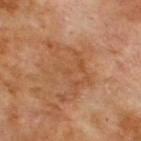workup: catalogued during a skin exam; not biopsied | anatomic site: the upper back | subject: male, in their 70s | size: ≈7 mm | lighting: cross-polarized | image source: 15 mm crop, total-body photography.